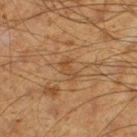Q: Was a biopsy performed?
A: total-body-photography surveillance lesion; no biopsy
Q: How was this image acquired?
A: total-body-photography crop, ~15 mm field of view
Q: Where on the body is the lesion?
A: the left thigh
Q: What are the patient's age and sex?
A: male, aged approximately 60